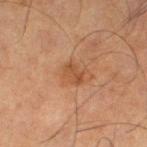No biopsy was performed on this lesion — it was imaged during a full skin examination and was not determined to be concerning. The tile uses cross-polarized illumination. On the left thigh. A male patient, in their mid-60s. An algorithmic analysis of the crop reported an eccentricity of roughly 0.75 and two-axis asymmetry of about 0.25. And it measured an average lesion color of about L≈41 a*≈20 b*≈31 (CIELAB), about 7 CIELAB-L* units darker than the surrounding skin, and a normalized lesion–skin contrast near 6.5. It also reported a border-irregularity rating of about 3/10 and radial color variation of about 0.5. Longest diameter approximately 2.5 mm. A 15 mm crop from a total-body photograph taken for skin-cancer surveillance.